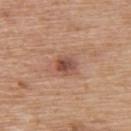Clinical summary:
The lesion's longest dimension is about 2.5 mm. On the upper back. Automated tile analysis of the lesion measured a footprint of about 5 mm², a shape eccentricity near 0.5, and a symmetry-axis asymmetry near 0.15. The analysis additionally found roughly 11 lightness units darker than nearby skin and a normalized lesion–skin contrast near 8. It also reported a nevus-likeness score of about 75/100 and a detector confidence of about 100 out of 100 that the crop contains a lesion. A lesion tile, about 15 mm wide, cut from a 3D total-body photograph. A male subject, aged around 60.